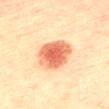biopsy status=total-body-photography surveillance lesion; no biopsy | patient=female, aged 63 to 67 | diameter=≈5.5 mm | image=~15 mm crop, total-body skin-cancer survey | TBP lesion metrics=a lesion color around L≈59 a*≈27 b*≈35 in CIELAB; border irregularity of about 2.5 on a 0–10 scale and a within-lesion color-variation index near 4.5/10; a classifier nevus-likeness of about 100/100 and a detector confidence of about 100 out of 100 that the crop contains a lesion | illumination=cross-polarized | location=the mid back.